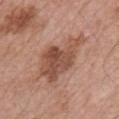The lesion was tiled from a total-body skin photograph and was not biopsied.
From the chest.
Imaged with white-light lighting.
The patient is a male aged around 65.
Cropped from a total-body skin-imaging series; the visible field is about 15 mm.